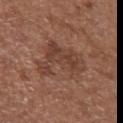biopsy_status: not biopsied; imaged during a skin examination
image:
  source: total-body photography crop
  field_of_view_mm: 15
site: front of the torso
patient:
  sex: female
  age_approx: 75
lesion_size:
  long_diameter_mm_approx: 6.5